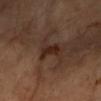<case>
  <biopsy_status>not biopsied; imaged during a skin examination</biopsy_status>
  <patient>
    <sex>female</sex>
    <age_approx>60</age_approx>
  </patient>
  <lesion_size>
    <long_diameter_mm_approx>3.0</long_diameter_mm_approx>
  </lesion_size>
  <lighting>cross-polarized</lighting>
  <site>left forearm</site>
  <image>
    <source>total-body photography crop</source>
    <field_of_view_mm>15</field_of_view_mm>
  </image>
</case>